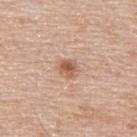Assessment: This lesion was catalogued during total-body skin photography and was not selected for biopsy. Context: The subject is a male aged approximately 55. Automated image analysis of the tile measured an area of roughly 4.5 mm² and an outline eccentricity of about 0.55 (0 = round, 1 = elongated). It also reported an average lesion color of about L≈59 a*≈21 b*≈32 (CIELAB), about 11 CIELAB-L* units darker than the surrounding skin, and a lesion-to-skin contrast of about 7.5 (normalized; higher = more distinct). And it measured a color-variation rating of about 5/10 and a peripheral color-asymmetry measure near 1.5. A close-up tile cropped from a whole-body skin photograph, about 15 mm across. The lesion is located on the upper back. Captured under white-light illumination. Measured at roughly 2.5 mm in maximum diameter.